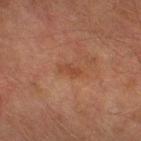Q: Is there a histopathology result?
A: catalogued during a skin exam; not biopsied
Q: Who is the patient?
A: male, in their mid- to late 70s
Q: How was this image acquired?
A: 15 mm crop, total-body photography
Q: Illumination type?
A: cross-polarized illumination
Q: Lesion size?
A: ~2.5 mm (longest diameter)
Q: What is the anatomic site?
A: the right thigh